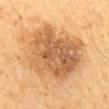Case summary:
• notes: total-body-photography surveillance lesion; no biopsy
• image source: 15 mm crop, total-body photography
• anatomic site: the mid back
• patient: male, roughly 60 years of age